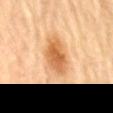Assessment: The lesion was photographed on a routine skin check and not biopsied; there is no pathology result. Clinical summary: From the mid back. A female patient, aged 78 to 82. Imaged with cross-polarized lighting. The lesion-visualizer software estimated a mean CIELAB color near L≈55 a*≈21 b*≈38 and a lesion-to-skin contrast of about 8.5 (normalized; higher = more distinct). And it measured internal color variation of about 5 on a 0–10 scale and a peripheral color-asymmetry measure near 1.5. A lesion tile, about 15 mm wide, cut from a 3D total-body photograph. The recorded lesion diameter is about 6 mm.